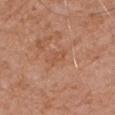Notes:
• follow-up: total-body-photography surveillance lesion; no biopsy
• tile lighting: white-light
• anatomic site: the left upper arm
• subject: male, aged 78–82
• lesion size: ≈2.5 mm
• image source: ~15 mm tile from a whole-body skin photo